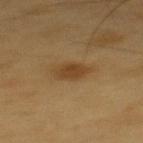workup = imaged on a skin check; not biopsied | imaging modality = ~15 mm tile from a whole-body skin photo | size = ≈4 mm | patient = male, aged 58 to 62 | lighting = cross-polarized | automated metrics = an area of roughly 6.5 mm², an eccentricity of roughly 0.8, and two-axis asymmetry of about 0.25; a border-irregularity index near 2.5/10, internal color variation of about 2 on a 0–10 scale, and radial color variation of about 0.5 | anatomic site = the upper back.